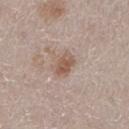notes = catalogued during a skin exam; not biopsied | automated metrics = a lesion color around L≈54 a*≈18 b*≈27 in CIELAB; an automated nevus-likeness rating near 55 out of 100 and lesion-presence confidence of about 100/100 | size = ~3 mm (longest diameter) | subject = female, aged around 50 | image = total-body-photography crop, ~15 mm field of view | site = the right lower leg | lighting = white-light illumination.